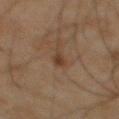  biopsy_status: not biopsied; imaged during a skin examination
  image:
    source: total-body photography crop
    field_of_view_mm: 15
  patient:
    sex: male
    age_approx: 65
  site: front of the torso